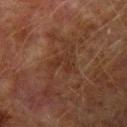The lesion was photographed on a routine skin check and not biopsied; there is no pathology result. Longest diameter approximately 3 mm. The lesion-visualizer software estimated an area of roughly 4 mm², an eccentricity of roughly 0.65, and two-axis asymmetry of about 0.6. And it measured border irregularity of about 6.5 on a 0–10 scale, a within-lesion color-variation index near 0/10, and a peripheral color-asymmetry measure near 0. The patient is a male aged around 75. A 15 mm close-up tile from a total-body photography series done for melanoma screening. Imaged with cross-polarized lighting. The lesion is located on the arm.